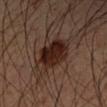• workup: imaged on a skin check; not biopsied
• tile lighting: cross-polarized
• patient: male, approximately 50 years of age
• body site: the right upper arm
• image: ~15 mm crop, total-body skin-cancer survey